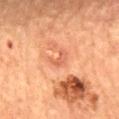Assessment: No biopsy was performed on this lesion — it was imaged during a full skin examination and was not determined to be concerning. Context: The total-body-photography lesion software estimated an average lesion color of about L≈52 a*≈27 b*≈32 (CIELAB). A female patient roughly 70 years of age. Cropped from a whole-body photographic skin survey; the tile spans about 15 mm. From the mid back.